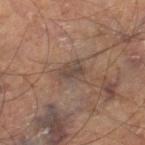| field | value |
|---|---|
| biopsy status | no biopsy performed (imaged during a skin exam) |
| lighting | cross-polarized |
| imaging modality | total-body-photography crop, ~15 mm field of view |
| automated lesion analysis | a lesion color around L≈46 a*≈14 b*≈23 in CIELAB and roughly 8 lightness units darker than nearby skin |
| lesion size | ≈3.5 mm |
| site | the right thigh |